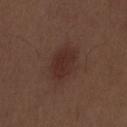Assessment: No biopsy was performed on this lesion — it was imaged during a full skin examination and was not determined to be concerning. Acquisition and patient details: A 15 mm crop from a total-body photograph taken for skin-cancer surveillance. Captured under white-light illumination. The lesion is on the front of the torso. A male patient aged 68–72.